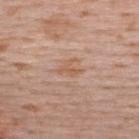Acquisition and patient details:
Located on the upper back. A male subject, aged 38 to 42. This is a white-light tile. This image is a 15 mm lesion crop taken from a total-body photograph.